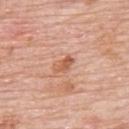  biopsy_status: not biopsied; imaged during a skin examination